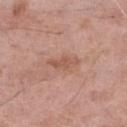This lesion was catalogued during total-body skin photography and was not selected for biopsy. This is a white-light tile. From the right thigh. A roughly 15 mm field-of-view crop from a total-body skin photograph. The subject is a female about 60 years old. An algorithmic analysis of the crop reported an average lesion color of about L≈55 a*≈22 b*≈29 (CIELAB) and a normalized border contrast of about 6. It also reported a border-irregularity index near 4.5/10 and a color-variation rating of about 1/10. Longest diameter approximately 3.5 mm.